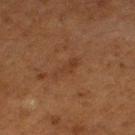Assessment: The lesion was photographed on a routine skin check and not biopsied; there is no pathology result. Context: A 15 mm close-up tile from a total-body photography series done for melanoma screening. A female subject, aged approximately 50. The lesion is on the left thigh.